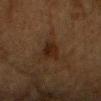Part of a total-body skin-imaging series; this lesion was reviewed on a skin check and was not flagged for biopsy.
A 15 mm crop from a total-body photograph taken for skin-cancer surveillance.
The lesion-visualizer software estimated a lesion area of about 4.5 mm² and an outline eccentricity of about 0.7 (0 = round, 1 = elongated). And it measured border irregularity of about 2.5 on a 0–10 scale, a within-lesion color-variation index near 3.5/10, and a peripheral color-asymmetry measure near 1. And it measured a nevus-likeness score of about 35/100 and a detector confidence of about 100 out of 100 that the crop contains a lesion.
The lesion is located on the chest.
This is a cross-polarized tile.
The recorded lesion diameter is about 3 mm.
A male patient, aged 73 to 77.